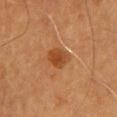Case summary:
- workup — catalogued during a skin exam; not biopsied
- subject — female, aged 58–62
- image source — ~15 mm tile from a whole-body skin photo
- body site — the upper back
- lesion diameter — ~3 mm (longest diameter)
- lighting — cross-polarized
- automated lesion analysis — a footprint of about 5.5 mm², a shape eccentricity near 0.5, and two-axis asymmetry of about 0.15; a border-irregularity index near 1.5/10, a within-lesion color-variation index near 3/10, and a peripheral color-asymmetry measure near 1; an automated nevus-likeness rating near 90 out of 100